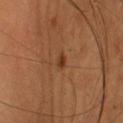Impression: The lesion was photographed on a routine skin check and not biopsied; there is no pathology result. Context: A lesion tile, about 15 mm wide, cut from a 3D total-body photograph. Automated tile analysis of the lesion measured an area of roughly 1.5 mm², a shape eccentricity near 0.75, and a shape-asymmetry score of about 0.25 (0 = symmetric). It also reported an average lesion color of about L≈36 a*≈24 b*≈33 (CIELAB). It also reported a border-irregularity index near 1.5/10, internal color variation of about 0 on a 0–10 scale, and peripheral color asymmetry of about 0. A female patient, roughly 35 years of age. On the head or neck. This is a cross-polarized tile.